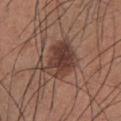Captured during whole-body skin photography for melanoma surveillance; the lesion was not biopsied.
The patient is a male aged approximately 35.
A 15 mm close-up tile from a total-body photography series done for melanoma screening.
Located on the chest.